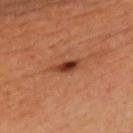Findings:
• biopsy status · imaged on a skin check; not biopsied
• image · total-body-photography crop, ~15 mm field of view
• body site · the chest
• illumination · cross-polarized illumination
• subject · male, roughly 45 years of age